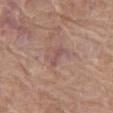notes: imaged on a skin check; not biopsied
automated lesion analysis: a footprint of about 3 mm², an outline eccentricity of about 0.9 (0 = round, 1 = elongated), and a symmetry-axis asymmetry near 0.35; a lesion-to-skin contrast of about 5.5 (normalized; higher = more distinct); lesion-presence confidence of about 90/100
subject: female, in their mid-70s
acquisition: ~15 mm tile from a whole-body skin photo
body site: the right thigh